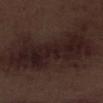| field | value |
|---|---|
| workup | total-body-photography surveillance lesion; no biopsy |
| lesion diameter | ≈13.5 mm |
| body site | the right lower leg |
| image | ~15 mm crop, total-body skin-cancer survey |
| subject | male, aged around 70 |